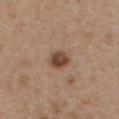Clinical impression:
Recorded during total-body skin imaging; not selected for excision or biopsy.
Clinical summary:
A male patient, about 50 years old. The lesion's longest dimension is about 3 mm. An algorithmic analysis of the crop reported a footprint of about 5.5 mm² and a symmetry-axis asymmetry near 0.15. And it measured a nevus-likeness score of about 95/100 and lesion-presence confidence of about 100/100. A 15 mm close-up tile from a total-body photography series done for melanoma screening. The lesion is on the front of the torso.